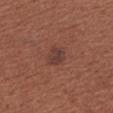Part of a total-body skin-imaging series; this lesion was reviewed on a skin check and was not flagged for biopsy.
A region of skin cropped from a whole-body photographic capture, roughly 15 mm wide.
A female patient, approximately 50 years of age.
On the arm.